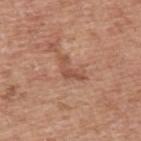Captured during whole-body skin photography for melanoma surveillance; the lesion was not biopsied. A region of skin cropped from a whole-body photographic capture, roughly 15 mm wide. The lesion is located on the upper back. A male subject, in their mid-50s. Imaged with white-light lighting.